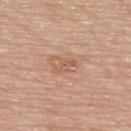workup = catalogued during a skin exam; not biopsied
subject = male, aged approximately 75
body site = the upper back
image source = total-body-photography crop, ~15 mm field of view
lighting = white-light illumination